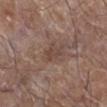{
  "biopsy_status": "not biopsied; imaged during a skin examination",
  "automated_metrics": {
    "area_mm2_approx": 4.0,
    "eccentricity": 0.8,
    "border_irregularity_0_10": 4.0,
    "color_variation_0_10": 2.0,
    "peripheral_color_asymmetry": 0.5
  },
  "lesion_size": {
    "long_diameter_mm_approx": 3.0
  },
  "site": "leg",
  "image": {
    "source": "total-body photography crop",
    "field_of_view_mm": 15
  },
  "patient": {
    "sex": "male",
    "age_approx": 65
  }
}